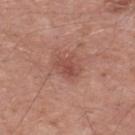Clinical impression: The lesion was photographed on a routine skin check and not biopsied; there is no pathology result. Background: The total-body-photography lesion software estimated an area of roughly 5 mm², an eccentricity of roughly 0.75, and two-axis asymmetry of about 0.25. And it measured a border-irregularity rating of about 3/10, a within-lesion color-variation index near 2.5/10, and peripheral color asymmetry of about 1. A 15 mm close-up tile from a total-body photography series done for melanoma screening. Imaged with white-light lighting. A male subject, aged approximately 55. Located on the mid back.